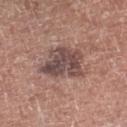Q: Was a biopsy performed?
A: no biopsy performed (imaged during a skin exam)
Q: What kind of image is this?
A: total-body-photography crop, ~15 mm field of view
Q: Lesion location?
A: the right lower leg
Q: Lesion size?
A: ≈6 mm
Q: What are the patient's age and sex?
A: male, aged 63–67